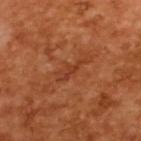Part of a total-body skin-imaging series; this lesion was reviewed on a skin check and was not flagged for biopsy. A male subject, in their mid-60s. This image is a 15 mm lesion crop taken from a total-body photograph. The recorded lesion diameter is about 4 mm. This is a cross-polarized tile.